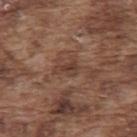Case summary:
– subject — male, approximately 75 years of age
– location — the upper back
– diameter — ≈3 mm
– image — ~15 mm crop, total-body skin-cancer survey
– image-analysis metrics — a lesion color around L≈39 a*≈19 b*≈25 in CIELAB, about 8 CIELAB-L* units darker than the surrounding skin, and a normalized border contrast of about 7; a nevus-likeness score of about 5/100 and a detector confidence of about 90 out of 100 that the crop contains a lesion